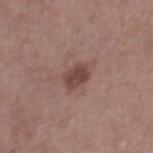{
  "patient": {
    "sex": "female",
    "age_approx": 40
  },
  "image": {
    "source": "total-body photography crop",
    "field_of_view_mm": 15
  },
  "site": "right thigh"
}